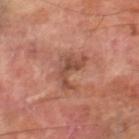About 4.5 mm across.
Located on the right lower leg.
Captured under cross-polarized illumination.
This image is a 15 mm lesion crop taken from a total-body photograph.
The patient is a male about 70 years old.